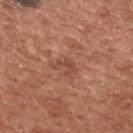Clinical impression:
Captured during whole-body skin photography for melanoma surveillance; the lesion was not biopsied.
Context:
Located on the upper back. A male patient aged around 55. A 15 mm close-up extracted from a 3D total-body photography capture.